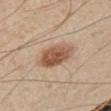| feature | finding |
|---|---|
| biopsy status | imaged on a skin check; not biopsied |
| acquisition | 15 mm crop, total-body photography |
| tile lighting | cross-polarized |
| body site | the arm |
| lesion diameter | about 4 mm |
| subject | male, aged 48 to 52 |
| image-analysis metrics | an average lesion color of about L≈43 a*≈16 b*≈25 (CIELAB), a lesion–skin lightness drop of about 11, and a normalized lesion–skin contrast near 9; a border-irregularity index near 1/10 and a peripheral color-asymmetry measure near 1.5 |